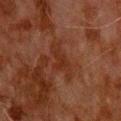Captured during whole-body skin photography for melanoma surveillance; the lesion was not biopsied.
A lesion tile, about 15 mm wide, cut from a 3D total-body photograph.
Approximately 4.5 mm at its widest.
A male subject, roughly 80 years of age.
The lesion is located on the upper back.